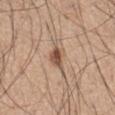Located on the abdomen. Approximately 3 mm at its widest. A male subject, aged approximately 70. A 15 mm close-up extracted from a 3D total-body photography capture. Automated image analysis of the tile measured a footprint of about 5 mm², a shape eccentricity near 0.8, and two-axis asymmetry of about 0.3. And it measured a within-lesion color-variation index near 4.5/10 and peripheral color asymmetry of about 1.5. It also reported a lesion-detection confidence of about 100/100. The tile uses white-light illumination.